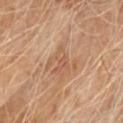{
  "biopsy_status": "not biopsied; imaged during a skin examination",
  "automated_metrics": {
    "area_mm2_approx": 4.5,
    "eccentricity": 0.85,
    "cielab_L": 55,
    "cielab_a": 22,
    "cielab_b": 32,
    "vs_skin_darker_L": 7.0,
    "vs_skin_contrast_norm": 5.0,
    "border_irregularity_0_10": 6.0,
    "color_variation_0_10": 3.0,
    "peripheral_color_asymmetry": 0.5
  },
  "patient": {
    "sex": "male",
    "age_approx": 70
  },
  "lighting": "cross-polarized",
  "site": "chest",
  "image": {
    "source": "total-body photography crop",
    "field_of_view_mm": 15
  },
  "lesion_size": {
    "long_diameter_mm_approx": 3.5
  }
}